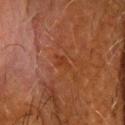workup: catalogued during a skin exam; not biopsied | illumination: cross-polarized illumination | automated metrics: an area of roughly 2.5 mm², an outline eccentricity of about 0.85 (0 = round, 1 = elongated), and a shape-asymmetry score of about 0.35 (0 = symmetric); a border-irregularity index near 4/10 and internal color variation of about 0 on a 0–10 scale; a classifier nevus-likeness of about 0/100 and a detector confidence of about 100 out of 100 that the crop contains a lesion | patient: male, about 70 years old | lesion size: ~2.5 mm (longest diameter) | site: the head or neck | image: ~15 mm tile from a whole-body skin photo.